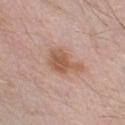Impression: This lesion was catalogued during total-body skin photography and was not selected for biopsy. Context: Longest diameter approximately 4.5 mm. Cropped from a total-body skin-imaging series; the visible field is about 15 mm. The lesion is located on the chest. Captured under white-light illumination. The subject is a male in their 30s.